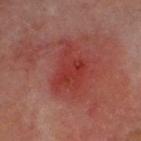No biopsy was performed on this lesion — it was imaged during a full skin examination and was not determined to be concerning. About 5.5 mm across. Imaged with cross-polarized lighting. Cropped from a whole-body photographic skin survey; the tile spans about 15 mm. From the head or neck. The subject is a male approximately 60 years of age. An algorithmic analysis of the crop reported a lesion color around L≈34 a*≈32 b*≈26 in CIELAB, roughly 6 lightness units darker than nearby skin, and a normalized border contrast of about 6.5. And it measured a border-irregularity rating of about 5.5/10, a color-variation rating of about 4/10, and peripheral color asymmetry of about 1.5. It also reported a nevus-likeness score of about 0/100 and a detector confidence of about 100 out of 100 that the crop contains a lesion.